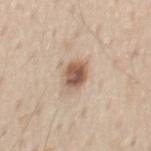Background:
An algorithmic analysis of the crop reported roughly 15 lightness units darker than nearby skin and a normalized border contrast of about 10. And it measured an automated nevus-likeness rating near 95 out of 100. The recorded lesion diameter is about 3 mm. A male subject about 60 years old. A 15 mm close-up extracted from a 3D total-body photography capture. Imaged with white-light lighting. The lesion is on the mid back.